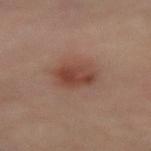biopsy status = imaged on a skin check; not biopsied | location = the lower back | lighting = cross-polarized | subject = female, roughly 50 years of age | imaging modality = ~15 mm tile from a whole-body skin photo | lesion diameter = ≈4 mm.